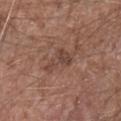  biopsy_status: not biopsied; imaged during a skin examination
  patient:
    sex: male
    age_approx: 70
  automated_metrics:
    area_mm2_approx: 7.0
    eccentricity: 0.85
    cielab_L: 44
    cielab_a: 19
    cielab_b: 25
    vs_skin_darker_L: 7.0
  site: arm
  image:
    source: total-body photography crop
    field_of_view_mm: 15
  lighting: white-light